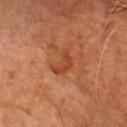This lesion was catalogued during total-body skin photography and was not selected for biopsy. Cropped from a whole-body photographic skin survey; the tile spans about 15 mm. On the chest. A male subject in their 80s. Automated image analysis of the tile measured an area of roughly 4.5 mm², a shape eccentricity near 0.8, and two-axis asymmetry of about 0.5. The analysis additionally found border irregularity of about 4.5 on a 0–10 scale and a color-variation rating of about 2/10. And it measured an automated nevus-likeness rating near 0 out of 100 and lesion-presence confidence of about 100/100. Approximately 3.5 mm at its widest.